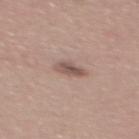The lesion was tiled from a total-body skin photograph and was not biopsied. Imaged with white-light lighting. The subject is a male aged around 40. An algorithmic analysis of the crop reported an outline eccentricity of about 0.75 (0 = round, 1 = elongated). The analysis additionally found a color-variation rating of about 4/10 and peripheral color asymmetry of about 1.5. Cropped from a whole-body photographic skin survey; the tile spans about 15 mm. On the back.